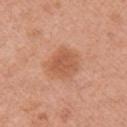Clinical impression: Captured during whole-body skin photography for melanoma surveillance; the lesion was not biopsied. Context: A female patient, aged 48–52. The lesion is located on the arm. A close-up tile cropped from a whole-body skin photograph, about 15 mm across.